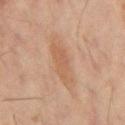{"biopsy_status": "not biopsied; imaged during a skin examination", "lesion_size": {"long_diameter_mm_approx": 6.0}, "site": "mid back", "patient": {"sex": "male", "age_approx": 60}, "image": {"source": "total-body photography crop", "field_of_view_mm": 15}, "automated_metrics": {"color_variation_0_10": 2.5, "peripheral_color_asymmetry": 1.0, "nevus_likeness_0_100": 0, "lesion_detection_confidence_0_100": 100}}